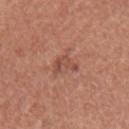This lesion was catalogued during total-body skin photography and was not selected for biopsy. A close-up tile cropped from a whole-body skin photograph, about 15 mm across. The subject is a female aged approximately 50. Imaged with white-light lighting. Located on the arm. Approximately 3 mm at its widest.